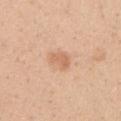Assessment:
Recorded during total-body skin imaging; not selected for excision or biopsy.
Image and clinical context:
The lesion is located on the right upper arm. A female subject aged around 25. The lesion-visualizer software estimated an area of roughly 4.5 mm², an outline eccentricity of about 0.75 (0 = round, 1 = elongated), and a shape-asymmetry score of about 0.35 (0 = symmetric). The software also gave a border-irregularity index near 3/10. And it measured a classifier nevus-likeness of about 90/100 and lesion-presence confidence of about 100/100. The lesion's longest dimension is about 3 mm. Cropped from a total-body skin-imaging series; the visible field is about 15 mm. Imaged with white-light lighting.